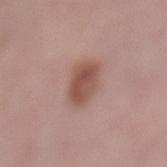follow-up: total-body-photography surveillance lesion; no biopsy
lighting: white-light illumination
location: the left lower leg
image-analysis metrics: a footprint of about 9.5 mm², an outline eccentricity of about 0.55 (0 = round, 1 = elongated), and two-axis asymmetry of about 0.2; an average lesion color of about L≈51 a*≈22 b*≈25 (CIELAB), a lesion–skin lightness drop of about 11, and a lesion-to-skin contrast of about 8 (normalized; higher = more distinct); a nevus-likeness score of about 100/100 and lesion-presence confidence of about 100/100
image source: 15 mm crop, total-body photography
subject: female, aged 48 to 52
size: about 4 mm